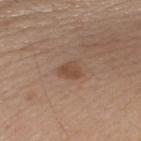biopsy_status: not biopsied; imaged during a skin examination